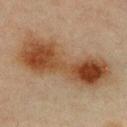Assessment: Captured during whole-body skin photography for melanoma surveillance; the lesion was not biopsied. Background: An algorithmic analysis of the crop reported a within-lesion color-variation index near 9/10 and radial color variation of about 3. The lesion is located on the chest. Approximately 12 mm at its widest. Captured under cross-polarized illumination. A 15 mm close-up extracted from a 3D total-body photography capture. A female subject aged 43–47.